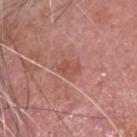Q: Was this lesion biopsied?
A: no biopsy performed (imaged during a skin exam)
Q: What is the anatomic site?
A: the head or neck
Q: What lighting was used for the tile?
A: white-light
Q: Automated lesion metrics?
A: an area of roughly 4 mm², an eccentricity of roughly 0.65, and a shape-asymmetry score of about 0.2 (0 = symmetric); roughly 7 lightness units darker than nearby skin and a lesion-to-skin contrast of about 5.5 (normalized; higher = more distinct); a border-irregularity index near 3/10 and a peripheral color-asymmetry measure near 1; an automated nevus-likeness rating near 0 out of 100 and a lesion-detection confidence of about 100/100
Q: How large is the lesion?
A: ≈2.5 mm
Q: How was this image acquired?
A: total-body-photography crop, ~15 mm field of view
Q: Patient demographics?
A: male, roughly 75 years of age